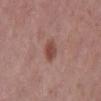  biopsy_status: not biopsied; imaged during a skin examination
  automated_metrics:
    area_mm2_approx: 5.0
    eccentricity: 0.55
    shape_asymmetry: 0.2
  patient:
    sex: male
    age_approx: 60
  site: back
  image:
    source: total-body photography crop
    field_of_view_mm: 15
  lighting: white-light
  lesion_size:
    long_diameter_mm_approx: 2.5